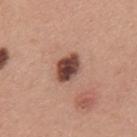Clinical impression:
The lesion was tiled from a total-body skin photograph and was not biopsied.
Clinical summary:
Automated image analysis of the tile measured a footprint of about 7 mm² and a shape-asymmetry score of about 0.15 (0 = symmetric). The analysis additionally found a mean CIELAB color near L≈44 a*≈22 b*≈25 and a normalized lesion–skin contrast near 13.5. The software also gave a border-irregularity index near 1.5/10 and radial color variation of about 1.5. Imaged with white-light lighting. A male patient aged approximately 40. From the upper back. Cropped from a total-body skin-imaging series; the visible field is about 15 mm. About 3.5 mm across.